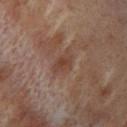Case summary:
- follow-up — total-body-photography surveillance lesion; no biopsy
- subject — female, aged 48 to 52
- image — ~15 mm crop, total-body skin-cancer survey
- lesion size — about 3 mm
- lighting — cross-polarized illumination
- anatomic site — the left lower leg
- TBP lesion metrics — border irregularity of about 3.5 on a 0–10 scale, a within-lesion color-variation index near 2/10, and radial color variation of about 0.5; a nevus-likeness score of about 0/100